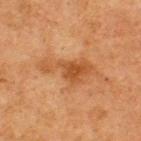– notes — catalogued during a skin exam; not biopsied
– illumination — cross-polarized illumination
– diameter — ≈6.5 mm
– subject — male, aged around 75
– TBP lesion metrics — a mean CIELAB color near L≈42 a*≈21 b*≈34, about 7 CIELAB-L* units darker than the surrounding skin, and a normalized border contrast of about 6.5
– acquisition — 15 mm crop, total-body photography
– anatomic site — the back